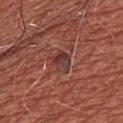Recorded during total-body skin imaging; not selected for excision or biopsy. The subject is a male in their mid-60s. A 15 mm close-up extracted from a 3D total-body photography capture. From the chest. Automated tile analysis of the lesion measured an average lesion color of about L≈37 a*≈22 b*≈24 (CIELAB), about 8 CIELAB-L* units darker than the surrounding skin, and a normalized border contrast of about 7.5. The analysis additionally found a border-irregularity rating of about 3.5/10, internal color variation of about 4.5 on a 0–10 scale, and peripheral color asymmetry of about 1.5. Measured at roughly 2.5 mm in maximum diameter.